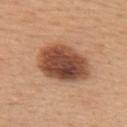<tbp_lesion>
<patient>
  <sex>female</sex>
  <age_approx>30</age_approx>
</patient>
<lesion_size>
  <long_diameter_mm_approx>6.5</long_diameter_mm_approx>
</lesion_size>
<image>
  <source>total-body photography crop</source>
  <field_of_view_mm>15</field_of_view_mm>
</image>
<site>upper back</site>
<lighting>white-light</lighting>
</tbp_lesion>